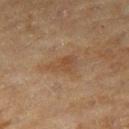Clinical impression: This lesion was catalogued during total-body skin photography and was not selected for biopsy. Clinical summary: The recorded lesion diameter is about 4 mm. Imaged with cross-polarized lighting. A 15 mm close-up tile from a total-body photography series done for melanoma screening. The total-body-photography lesion software estimated an area of roughly 6 mm², an outline eccentricity of about 0.8 (0 = round, 1 = elongated), and a shape-asymmetry score of about 0.4 (0 = symmetric). The analysis additionally found a lesion color around L≈43 a*≈17 b*≈30 in CIELAB, about 6 CIELAB-L* units darker than the surrounding skin, and a lesion-to-skin contrast of about 6 (normalized; higher = more distinct). And it measured border irregularity of about 4.5 on a 0–10 scale, a color-variation rating of about 2.5/10, and peripheral color asymmetry of about 0.5. It also reported a classifier nevus-likeness of about 0/100 and a lesion-detection confidence of about 100/100. From the right thigh. A female patient roughly 60 years of age.